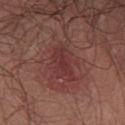The lesion was photographed on a routine skin check and not biopsied; there is no pathology result. Measured at roughly 5 mm in maximum diameter. The subject is a male about 55 years old. This is a white-light tile. A 15 mm close-up extracted from a 3D total-body photography capture. Located on the chest.